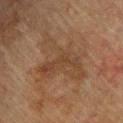Findings:
• biopsy status · catalogued during a skin exam; not biopsied
• size · about 8 mm
• patient · male, in their mid-70s
• imaging modality · 15 mm crop, total-body photography
• tile lighting · cross-polarized
• location · the back